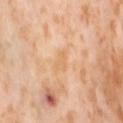No biopsy was performed on this lesion — it was imaged during a full skin examination and was not determined to be concerning. A lesion tile, about 15 mm wide, cut from a 3D total-body photograph. Captured under cross-polarized illumination. The lesion is located on the lower back. A female subject, aged 53–57. Automated tile analysis of the lesion measured a nevus-likeness score of about 0/100 and lesion-presence confidence of about 100/100. Measured at roughly 2.5 mm in maximum diameter.